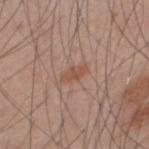<lesion>
  <biopsy_status>not biopsied; imaged during a skin examination</biopsy_status>
  <patient>
    <sex>male</sex>
    <age_approx>35</age_approx>
  </patient>
  <site>upper back</site>
  <image>
    <source>total-body photography crop</source>
    <field_of_view_mm>15</field_of_view_mm>
  </image>
  <lighting>white-light</lighting>
</lesion>